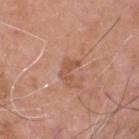Captured during whole-body skin photography for melanoma surveillance; the lesion was not biopsied.
Approximately 2.5 mm at its widest.
A roughly 15 mm field-of-view crop from a total-body skin photograph.
Automated image analysis of the tile measured two-axis asymmetry of about 0.55. And it measured a lesion color around L≈53 a*≈23 b*≈31 in CIELAB, a lesion–skin lightness drop of about 8, and a lesion-to-skin contrast of about 5.5 (normalized; higher = more distinct). The software also gave a border-irregularity index near 7/10, a color-variation rating of about 0/10, and radial color variation of about 0. The software also gave lesion-presence confidence of about 100/100.
Imaged with white-light lighting.
A male patient aged 48–52.
The lesion is located on the head or neck.